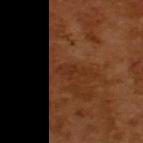Case summary:
• subject — male, aged 63 to 67
• lighting — cross-polarized
• size — ~3 mm (longest diameter)
• image-analysis metrics — a footprint of about 3 mm² and two-axis asymmetry of about 0.45; a lesion color around L≈29 a*≈24 b*≈31 in CIELAB and a lesion-to-skin contrast of about 5 (normalized; higher = more distinct); a border-irregularity index near 5.5/10 and a peripheral color-asymmetry measure near 0; an automated nevus-likeness rating near 0 out of 100
• acquisition — 15 mm crop, total-body photography